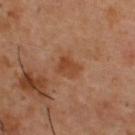Assessment:
Recorded during total-body skin imaging; not selected for excision or biopsy.
Context:
Cropped from a whole-body photographic skin survey; the tile spans about 15 mm. The lesion is on the upper back. A male subject, aged around 55.